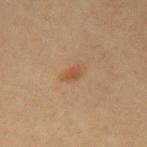<lesion>
<biopsy_status>not biopsied; imaged during a skin examination</biopsy_status>
<patient>
  <sex>female</sex>
  <age_approx>40</age_approx>
</patient>
<site>arm</site>
<automated_metrics>
  <cielab_L>43</cielab_L>
  <cielab_a>18</cielab_a>
  <cielab_b>30</cielab_b>
  <vs_skin_darker_L>7.0</vs_skin_darker_L>
  <vs_skin_contrast_norm>6.0</vs_skin_contrast_norm>
  <border_irregularity_0_10>2.0</border_irregularity_0_10>
  <peripheral_color_asymmetry>1.0</peripheral_color_asymmetry>
</automated_metrics>
<lighting>cross-polarized</lighting>
<lesion_size>
  <long_diameter_mm_approx>2.5</long_diameter_mm_approx>
</lesion_size>
<image>
  <source>total-body photography crop</source>
  <field_of_view_mm>15</field_of_view_mm>
</image>
</lesion>